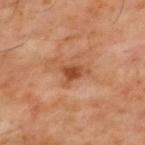Q: Is there a histopathology result?
A: imaged on a skin check; not biopsied
Q: What is the imaging modality?
A: ~15 mm tile from a whole-body skin photo
Q: Where on the body is the lesion?
A: the mid back
Q: What is the lesion's diameter?
A: ≈3.5 mm
Q: What are the patient's age and sex?
A: male, aged 58 to 62
Q: How was the tile lit?
A: cross-polarized illumination